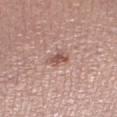illumination = white-light illumination; acquisition = 15 mm crop, total-body photography; TBP lesion metrics = a nevus-likeness score of about 55/100 and a detector confidence of about 100 out of 100 that the crop contains a lesion; subject = male, approximately 75 years of age; location = the right lower leg; size = ≈2.5 mm.